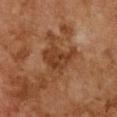Clinical impression: Part of a total-body skin-imaging series; this lesion was reviewed on a skin check and was not flagged for biopsy. Image and clinical context: From the chest. Cropped from a whole-body photographic skin survey; the tile spans about 15 mm. A female subject in their 60s.